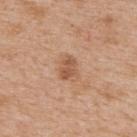Impression:
Part of a total-body skin-imaging series; this lesion was reviewed on a skin check and was not flagged for biopsy.
Background:
A male patient in their mid-60s. Longest diameter approximately 2.5 mm. Located on the back. A roughly 15 mm field-of-view crop from a total-body skin photograph. This is a white-light tile.